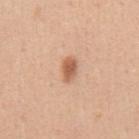biopsy_status: not biopsied; imaged during a skin examination
image:
  source: total-body photography crop
  field_of_view_mm: 15
lighting: white-light
lesion_size:
  long_diameter_mm_approx: 2.5
automated_metrics:
  area_mm2_approx: 4.0
  eccentricity: 0.8
  shape_asymmetry: 0.25
  border_irregularity_0_10: 2.5
  color_variation_0_10: 2.5
  peripheral_color_asymmetry: 0.5
site: front of the torso
patient:
  sex: male
  age_approx: 50